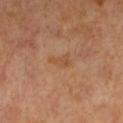Recorded during total-body skin imaging; not selected for excision or biopsy.
A 15 mm crop from a total-body photograph taken for skin-cancer surveillance.
Imaged with cross-polarized lighting.
The lesion is on the leg.
The lesion's longest dimension is about 3 mm.
A female patient, aged 53–57.